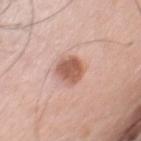Captured during whole-body skin photography for melanoma surveillance; the lesion was not biopsied.
This image is a 15 mm lesion crop taken from a total-body photograph.
Captured under white-light illumination.
Longest diameter approximately 3.5 mm.
A male subject, aged approximately 80.
The lesion is on the chest.